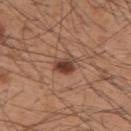{
  "biopsy_status": "not biopsied; imaged during a skin examination",
  "automated_metrics": {
    "eccentricity": 0.8,
    "shape_asymmetry": 0.25,
    "cielab_L": 42,
    "cielab_a": 21,
    "cielab_b": 28,
    "vs_skin_darker_L": 13.0,
    "vs_skin_contrast_norm": 10.0,
    "nevus_likeness_0_100": 95,
    "lesion_detection_confidence_0_100": 100
  },
  "patient": {
    "sex": "male",
    "age_approx": 60
  },
  "site": "upper back",
  "image": {
    "source": "total-body photography crop",
    "field_of_view_mm": 15
  }
}